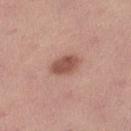{"lighting": "white-light", "patient": {"sex": "female", "age_approx": 25}, "image": {"source": "total-body photography crop", "field_of_view_mm": 15}, "lesion_size": {"long_diameter_mm_approx": 4.0}, "site": "left lower leg"}